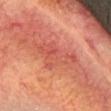<tbp_lesion>
  <biopsy_status>not biopsied; imaged during a skin examination</biopsy_status>
  <site>head or neck</site>
  <image>
    <source>total-body photography crop</source>
    <field_of_view_mm>15</field_of_view_mm>
  </image>
  <patient>
    <sex>female</sex>
    <age_approx>75</age_approx>
  </patient>
  <lesion_size>
    <long_diameter_mm_approx>6.5</long_diameter_mm_approx>
  </lesion_size>
  <lighting>cross-polarized</lighting>
  <automated_metrics>
    <area_mm2_approx>11.0</area_mm2_approx>
    <shape_asymmetry>0.5</shape_asymmetry>
  </automated_metrics>
</tbp_lesion>